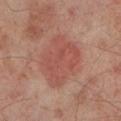biopsy status: total-body-photography surveillance lesion; no biopsy
lighting: cross-polarized
patient: male, roughly 50 years of age
TBP lesion metrics: a lesion area of about 24 mm², an outline eccentricity of about 0.7 (0 = round, 1 = elongated), and two-axis asymmetry of about 0.2; a mean CIELAB color near L≈50 a*≈26 b*≈26, about 7 CIELAB-L* units darker than the surrounding skin, and a normalized lesion–skin contrast near 5.5; a color-variation rating of about 3/10 and peripheral color asymmetry of about 1
size: about 6.5 mm
anatomic site: the leg
image source: 15 mm crop, total-body photography